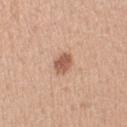diameter — about 3 mm
image source — ~15 mm crop, total-body skin-cancer survey
image-analysis metrics — a normalized lesion–skin contrast near 8.5; radial color variation of about 1
illumination — white-light illumination
patient — female, roughly 40 years of age
body site — the right upper arm